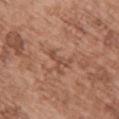The lesion was photographed on a routine skin check and not biopsied; there is no pathology result.